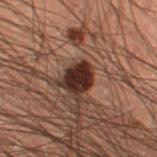lesion size = about 3.5 mm | lighting = cross-polarized illumination | patient = male, in their mid- to late 50s | location = the left thigh | image source = total-body-photography crop, ~15 mm field of view | automated metrics = an automated nevus-likeness rating near 95 out of 100 and a lesion-detection confidence of about 100/100.